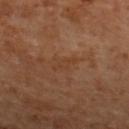Captured during whole-body skin photography for melanoma surveillance; the lesion was not biopsied.
The total-body-photography lesion software estimated a lesion area of about 2.5 mm² and two-axis asymmetry of about 0.3. And it measured roughly 4 lightness units darker than nearby skin and a normalized lesion–skin contrast near 4.5. And it measured a classifier nevus-likeness of about 0/100.
A roughly 15 mm field-of-view crop from a total-body skin photograph.
Approximately 3.5 mm at its widest.
The lesion is located on the back.
Captured under cross-polarized illumination.
The subject is a female aged 53–57.